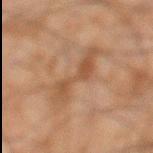notes — no biopsy performed (imaged during a skin exam) | site — the left lower leg | image — ~15 mm tile from a whole-body skin photo | patient — male, in their mid-40s | automated lesion analysis — an area of roughly 9 mm² and a shape-asymmetry score of about 0.4 (0 = symmetric); a classifier nevus-likeness of about 0/100 and a lesion-detection confidence of about 100/100 | tile lighting — cross-polarized.